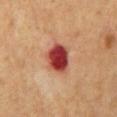Q: Was this lesion biopsied?
A: imaged on a skin check; not biopsied
Q: What is the lesion's diameter?
A: ~4 mm (longest diameter)
Q: Lesion location?
A: the mid back
Q: What is the imaging modality?
A: ~15 mm tile from a whole-body skin photo
Q: What did automated image analysis measure?
A: an average lesion color of about L≈39 a*≈32 b*≈27 (CIELAB), a lesion–skin lightness drop of about 18, and a normalized lesion–skin contrast near 14; a lesion-detection confidence of about 100/100
Q: Patient demographics?
A: male, aged around 60